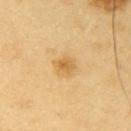follow-up: catalogued during a skin exam; not biopsied
illumination: cross-polarized illumination
subject: male, aged around 60
imaging modality: 15 mm crop, total-body photography
site: the right upper arm
image-analysis metrics: an average lesion color of about L≈63 a*≈17 b*≈45 (CIELAB), about 9 CIELAB-L* units darker than the surrounding skin, and a normalized lesion–skin contrast near 6.5